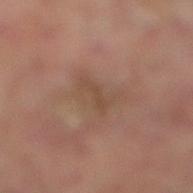About 3 mm across. On the right lower leg. This is a cross-polarized tile. Cropped from a total-body skin-imaging series; the visible field is about 15 mm. A male patient aged 63–67.